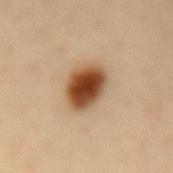follow-up: catalogued during a skin exam; not biopsied | site: the back | subject: female, aged 48 to 52 | lighting: cross-polarized illumination | acquisition: 15 mm crop, total-body photography | automated lesion analysis: an area of roughly 12 mm², an outline eccentricity of about 0.55 (0 = round, 1 = elongated), and two-axis asymmetry of about 0.1; a border-irregularity index near 1/10, internal color variation of about 6.5 on a 0–10 scale, and radial color variation of about 1.5.